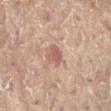biopsy status: imaged on a skin check; not biopsied
automated metrics: a lesion color around L≈51 a*≈22 b*≈22 in CIELAB, a lesion–skin lightness drop of about 9, and a normalized lesion–skin contrast near 7
size: ≈2.5 mm
imaging modality: total-body-photography crop, ~15 mm field of view
tile lighting: cross-polarized
body site: the left leg
subject: female, roughly 80 years of age